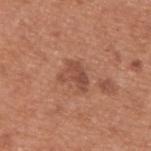Part of a total-body skin-imaging series; this lesion was reviewed on a skin check and was not flagged for biopsy.
A region of skin cropped from a whole-body photographic capture, roughly 15 mm wide.
A male subject aged around 55.
From the upper back.